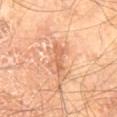{"biopsy_status": "not biopsied; imaged during a skin examination", "site": "right thigh", "patient": {"sex": "male", "age_approx": 70}, "automated_metrics": {"area_mm2_approx": 6.0, "shape_asymmetry": 0.45, "cielab_L": 64, "cielab_a": 25, "cielab_b": 37, "vs_skin_contrast_norm": 6.0, "border_irregularity_0_10": 6.0, "color_variation_0_10": 1.5, "peripheral_color_asymmetry": 0.5, "lesion_detection_confidence_0_100": 100}, "lesion_size": {"long_diameter_mm_approx": 4.5}, "image": {"source": "total-body photography crop", "field_of_view_mm": 15}}